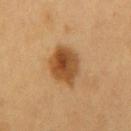acquisition = ~15 mm tile from a whole-body skin photo | subject = male, in their 60s | tile lighting = cross-polarized | anatomic site = the chest | size = about 5 mm.